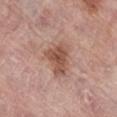The lesion was tiled from a total-body skin photograph and was not biopsied. Automated tile analysis of the lesion measured a border-irregularity rating of about 4/10 and radial color variation of about 1. The analysis additionally found a lesion-detection confidence of about 100/100. The subject is a female roughly 60 years of age. The recorded lesion diameter is about 4.5 mm. A lesion tile, about 15 mm wide, cut from a 3D total-body photograph. Imaged with white-light lighting. On the leg.